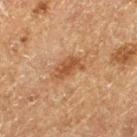Recorded during total-body skin imaging; not selected for excision or biopsy. The lesion is located on the left thigh. The tile uses cross-polarized illumination. A roughly 15 mm field-of-view crop from a total-body skin photograph. The subject is a male approximately 75 years of age. Automated image analysis of the tile measured a footprint of about 7.5 mm², an eccentricity of roughly 0.85, and a shape-asymmetry score of about 0.3 (0 = symmetric). The software also gave a lesion color around L≈42 a*≈19 b*≈31 in CIELAB, a lesion–skin lightness drop of about 8, and a lesion-to-skin contrast of about 7 (normalized; higher = more distinct). Measured at roughly 4.5 mm in maximum diameter.